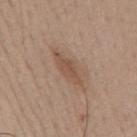Clinical impression: This lesion was catalogued during total-body skin photography and was not selected for biopsy. Image and clinical context: From the mid back. Automated tile analysis of the lesion measured a border-irregularity index near 3.5/10, internal color variation of about 2 on a 0–10 scale, and peripheral color asymmetry of about 0.5. And it measured an automated nevus-likeness rating near 15 out of 100 and a detector confidence of about 100 out of 100 that the crop contains a lesion. Captured under white-light illumination. A roughly 15 mm field-of-view crop from a total-body skin photograph. The lesion's longest dimension is about 5 mm. The subject is a male aged approximately 40.